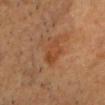The lesion was photographed on a routine skin check and not biopsied; there is no pathology result. A male subject, approximately 55 years of age. On the head or neck. The tile uses cross-polarized illumination. A 15 mm close-up tile from a total-body photography series done for melanoma screening. Measured at roughly 4 mm in maximum diameter.